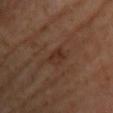Captured during whole-body skin photography for melanoma surveillance; the lesion was not biopsied.
A female patient aged around 60.
A 15 mm crop from a total-body photograph taken for skin-cancer surveillance.
The lesion's longest dimension is about 2.5 mm.
Located on the chest.
An algorithmic analysis of the crop reported border irregularity of about 3 on a 0–10 scale and radial color variation of about 0.5.